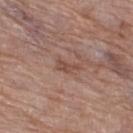biopsy status: catalogued during a skin exam; not biopsied
image: ~15 mm crop, total-body skin-cancer survey
lighting: white-light illumination
image-analysis metrics: a border-irregularity index near 5.5/10 and peripheral color asymmetry of about 0
anatomic site: the right thigh
patient: female, aged 68 to 72